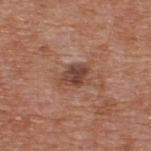<case>
  <biopsy_status>not biopsied; imaged during a skin examination</biopsy_status>
  <automated_metrics>
    <nevus_likeness_0_100>0</nevus_likeness_0_100>
  </automated_metrics>
  <patient>
    <sex>male</sex>
    <age_approx>65</age_approx>
  </patient>
  <image>
    <source>total-body photography crop</source>
    <field_of_view_mm>15</field_of_view_mm>
  </image>
  <site>upper back</site>
  <lesion_size>
    <long_diameter_mm_approx>3.0</long_diameter_mm_approx>
  </lesion_size>
</case>